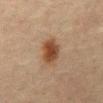The lesion was photographed on a routine skin check and not biopsied; there is no pathology result. About 4 mm across. A male patient aged around 65. The lesion is located on the abdomen. Cropped from a whole-body photographic skin survey; the tile spans about 15 mm. Captured under cross-polarized illumination. The lesion-visualizer software estimated a footprint of about 7.5 mm², a shape eccentricity near 0.75, and a symmetry-axis asymmetry near 0.2. The analysis additionally found a classifier nevus-likeness of about 100/100 and lesion-presence confidence of about 100/100.